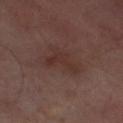workup: total-body-photography surveillance lesion; no biopsy | subject: male, roughly 65 years of age | image-analysis metrics: a peripheral color-asymmetry measure near 0.5 | tile lighting: cross-polarized | image source: ~15 mm tile from a whole-body skin photo | anatomic site: the leg.